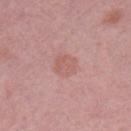  biopsy_status: not biopsied; imaged during a skin examination
  site: leg
  patient:
    sex: female
    age_approx: 50
  image:
    source: total-body photography crop
    field_of_view_mm: 15
  automated_metrics:
    border_irregularity_0_10: 1.5
    color_variation_0_10: 2.5
    peripheral_color_asymmetry: 1.0